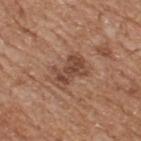{"biopsy_status": "not biopsied; imaged during a skin examination", "lesion_size": {"long_diameter_mm_approx": 4.0}, "image": {"source": "total-body photography crop", "field_of_view_mm": 15}, "patient": {"sex": "male", "age_approx": 65}, "site": "back", "lighting": "white-light"}